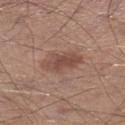biopsy status = no biopsy performed (imaged during a skin exam)
automated metrics = a mean CIELAB color near L≈48 a*≈19 b*≈25, roughly 9 lightness units darker than nearby skin, and a normalized border contrast of about 7
lesion diameter = ~5 mm (longest diameter)
anatomic site = the left lower leg
subject = male, aged around 30
image = ~15 mm crop, total-body skin-cancer survey